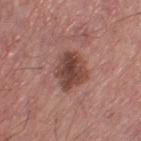| field | value |
|---|---|
| location | the left thigh |
| patient | male, aged 68–72 |
| tile lighting | white-light |
| image-analysis metrics | a lesion color around L≈44 a*≈22 b*≈24 in CIELAB and a normalized lesion–skin contrast near 9.5; a classifier nevus-likeness of about 50/100 and a detector confidence of about 100 out of 100 that the crop contains a lesion |
| image source | 15 mm crop, total-body photography |
| size | ~4 mm (longest diameter) |